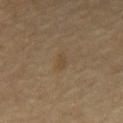Assessment:
No biopsy was performed on this lesion — it was imaged during a full skin examination and was not determined to be concerning.
Context:
Measured at roughly 2.5 mm in maximum diameter. On the chest. A male subject about 70 years old. A roughly 15 mm field-of-view crop from a total-body skin photograph.